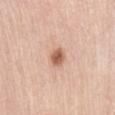Imaged during a routine full-body skin examination; the lesion was not biopsied and no histopathology is available. Approximately 2.5 mm at its widest. The lesion-visualizer software estimated a lesion area of about 4 mm², an outline eccentricity of about 0.7 (0 = round, 1 = elongated), and two-axis asymmetry of about 0.2. The software also gave a border-irregularity rating of about 1.5/10, a within-lesion color-variation index near 3/10, and radial color variation of about 1. It also reported a detector confidence of about 100 out of 100 that the crop contains a lesion. A roughly 15 mm field-of-view crop from a total-body skin photograph. A female patient roughly 65 years of age. On the abdomen.